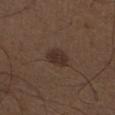Impression:
Imaged during a routine full-body skin examination; the lesion was not biopsied and no histopathology is available.
Background:
Measured at roughly 3 mm in maximum diameter. A close-up tile cropped from a whole-body skin photograph, about 15 mm across. A male subject in their 50s. From the left lower leg. Imaged with white-light lighting.